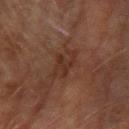This lesion was catalogued during total-body skin photography and was not selected for biopsy.
A male patient, aged 73–77.
The lesion is located on the left forearm.
A 15 mm crop from a total-body photograph taken for skin-cancer surveillance.
The recorded lesion diameter is about 3.5 mm.
Automated tile analysis of the lesion measured an area of roughly 6 mm², a shape eccentricity near 0.85, and two-axis asymmetry of about 0.4. It also reported roughly 5 lightness units darker than nearby skin and a lesion-to-skin contrast of about 6 (normalized; higher = more distinct). And it measured an automated nevus-likeness rating near 0 out of 100 and a detector confidence of about 100 out of 100 that the crop contains a lesion.
Imaged with cross-polarized lighting.